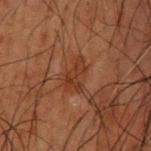Q: Was this lesion biopsied?
A: imaged on a skin check; not biopsied
Q: Where on the body is the lesion?
A: the upper back
Q: What are the patient's age and sex?
A: male, aged 48 to 52
Q: Illumination type?
A: cross-polarized
Q: What is the imaging modality?
A: 15 mm crop, total-body photography
Q: What is the lesion's diameter?
A: about 3.5 mm